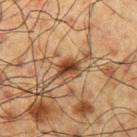notes: no biopsy performed (imaged during a skin exam).